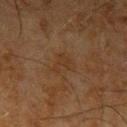notes: total-body-photography surveillance lesion; no biopsy
subject: male, about 65 years old
automated lesion analysis: two-axis asymmetry of about 0.6; border irregularity of about 6.5 on a 0–10 scale and a peripheral color-asymmetry measure near 0.5; a nevus-likeness score of about 0/100 and a detector confidence of about 100 out of 100 that the crop contains a lesion
imaging modality: ~15 mm tile from a whole-body skin photo
site: the left upper arm
lighting: cross-polarized
size: ≈3 mm